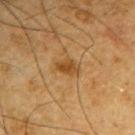location: the right upper arm | size: ~3 mm (longest diameter) | image source: ~15 mm crop, total-body skin-cancer survey | patient: male, in their mid- to late 60s | tile lighting: cross-polarized | image-analysis metrics: a footprint of about 4.5 mm² and a symmetry-axis asymmetry near 0.25; a border-irregularity rating of about 2.5/10 and peripheral color asymmetry of about 1; a classifier nevus-likeness of about 60/100 and a lesion-detection confidence of about 100/100.